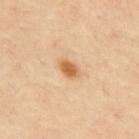  biopsy_status: not biopsied; imaged during a skin examination
  lesion_size:
    long_diameter_mm_approx: 2.5
  patient:
    sex: male
    age_approx: 55
  image:
    source: total-body photography crop
    field_of_view_mm: 15
  site: back